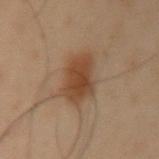The lesion was photographed on a routine skin check and not biopsied; there is no pathology result.
The subject is a male aged around 55.
A close-up tile cropped from a whole-body skin photograph, about 15 mm across.
The lesion is located on the left upper arm.